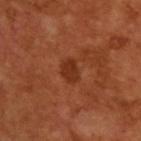- image source — 15 mm crop, total-body photography
- TBP lesion metrics — an average lesion color of about L≈32 a*≈28 b*≈33 (CIELAB), a lesion–skin lightness drop of about 8, and a lesion-to-skin contrast of about 7 (normalized; higher = more distinct); a lesion-detection confidence of about 100/100
- illumination — cross-polarized
- size — ≈2.5 mm
- patient — male, in their mid- to late 60s